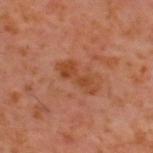| field | value |
|---|---|
| follow-up | catalogued during a skin exam; not biopsied |
| acquisition | 15 mm crop, total-body photography |
| anatomic site | the upper back |
| diameter | about 4.5 mm |
| subject | male, roughly 60 years of age |
| tile lighting | cross-polarized illumination |